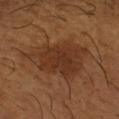This lesion was catalogued during total-body skin photography and was not selected for biopsy. A close-up tile cropped from a whole-body skin photograph, about 15 mm across. A male subject, roughly 65 years of age. The lesion is located on the left forearm.